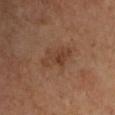workup: catalogued during a skin exam; not biopsied
tile lighting: cross-polarized illumination
size: ≈4 mm
automated metrics: a mean CIELAB color near L≈38 a*≈19 b*≈28 and a lesion-to-skin contrast of about 6.5 (normalized; higher = more distinct); a within-lesion color-variation index near 2.5/10
acquisition: ~15 mm tile from a whole-body skin photo
patient: male, about 65 years old
anatomic site: the left upper arm